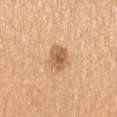Case summary:
– follow-up — no biopsy performed (imaged during a skin exam)
– subject — female, in their 60s
– body site — the left upper arm
– image — 15 mm crop, total-body photography